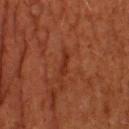Q: Was this lesion biopsied?
A: no biopsy performed (imaged during a skin exam)
Q: What are the patient's age and sex?
A: male, aged around 60
Q: Where on the body is the lesion?
A: the upper back
Q: What did automated image analysis measure?
A: an average lesion color of about L≈26 a*≈25 b*≈28 (CIELAB), roughly 6 lightness units darker than nearby skin, and a normalized lesion–skin contrast near 6; a border-irregularity rating of about 4.5/10 and a within-lesion color-variation index near 0/10; a classifier nevus-likeness of about 0/100 and a lesion-detection confidence of about 80/100
Q: What is the imaging modality?
A: 15 mm crop, total-body photography
Q: How was the tile lit?
A: cross-polarized